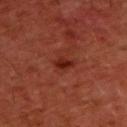Assessment:
Recorded during total-body skin imaging; not selected for excision or biopsy.
Image and clinical context:
Captured under cross-polarized illumination. A male subject roughly 60 years of age. The recorded lesion diameter is about 2 mm. A 15 mm close-up tile from a total-body photography series done for melanoma screening. The lesion is located on the back.